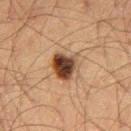Captured during whole-body skin photography for melanoma surveillance; the lesion was not biopsied. A 15 mm close-up tile from a total-body photography series done for melanoma screening. The lesion is on the left thigh. A male subject, in their mid-60s. Measured at roughly 3.5 mm in maximum diameter. Automated image analysis of the tile measured an average lesion color of about L≈36 a*≈18 b*≈27 (CIELAB) and a lesion–skin lightness drop of about 17. The tile uses cross-polarized illumination.